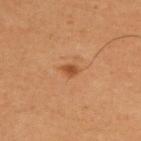biopsy status — catalogued during a skin exam; not biopsied | TBP lesion metrics — a footprint of about 2.5 mm² and a shape-asymmetry score of about 0.2 (0 = symmetric); a lesion-detection confidence of about 100/100 | lesion diameter — ≈2 mm | subject — male, aged 63 to 67 | tile lighting — cross-polarized illumination | site — the upper back | acquisition — total-body-photography crop, ~15 mm field of view.